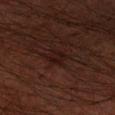Captured during whole-body skin photography for melanoma surveillance; the lesion was not biopsied.
Approximately 2.5 mm at its widest.
A male subject, about 60 years old.
The lesion is on the right forearm.
The lesion-visualizer software estimated a lesion area of about 4.5 mm², a shape eccentricity near 0.55, and two-axis asymmetry of about 0.25. And it measured a lesion color around L≈14 a*≈16 b*≈16 in CIELAB, roughly 5 lightness units darker than nearby skin, and a normalized border contrast of about 7. It also reported border irregularity of about 2.5 on a 0–10 scale. The analysis additionally found a classifier nevus-likeness of about 0/100.
A 15 mm close-up tile from a total-body photography series done for melanoma screening.